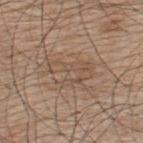workup: no biopsy performed (imaged during a skin exam) | lesion diameter: ≈5 mm | patient: male, aged approximately 75 | image: total-body-photography crop, ~15 mm field of view | location: the back | tile lighting: white-light illumination.